The lesion's longest dimension is about 10 mm. This image is a 15 mm lesion crop taken from a total-body photograph. A male patient about 55 years old. On the right forearm — 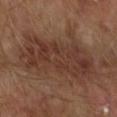diagnosis = a squamous cell carcinoma in situ.